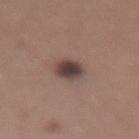Located on the leg. The recorded lesion diameter is about 3 mm. This is a white-light tile. A female patient about 30 years old. A 15 mm close-up extracted from a 3D total-body photography capture.